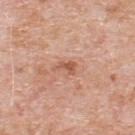| field | value |
|---|---|
| workup | imaged on a skin check; not biopsied |
| acquisition | ~15 mm crop, total-body skin-cancer survey |
| subject | male, aged 78 to 82 |
| anatomic site | the upper back |